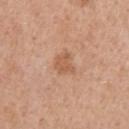{
  "biopsy_status": "not biopsied; imaged during a skin examination",
  "site": "right upper arm",
  "patient": {
    "sex": "female",
    "age_approx": 40
  },
  "lesion_size": {
    "long_diameter_mm_approx": 2.5
  },
  "image": {
    "source": "total-body photography crop",
    "field_of_view_mm": 15
  }
}